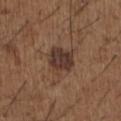Assessment: The lesion was tiled from a total-body skin photograph and was not biopsied. Context: Located on the left upper arm. A male subject about 50 years old. A close-up tile cropped from a whole-body skin photograph, about 15 mm across.